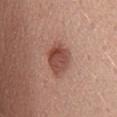Assessment: No biopsy was performed on this lesion — it was imaged during a full skin examination and was not determined to be concerning. Clinical summary: A female patient, aged approximately 35. Captured under white-light illumination. Approximately 4.5 mm at its widest. On the abdomen. The lesion-visualizer software estimated an area of roughly 9 mm² and a symmetry-axis asymmetry near 0.15. And it measured a nevus-likeness score of about 90/100. A 15 mm close-up extracted from a 3D total-body photography capture.